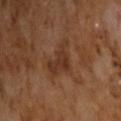workup=no biopsy performed (imaged during a skin exam) | diameter=~4 mm (longest diameter) | image-analysis metrics=a lesion area of about 7.5 mm²; a nevus-likeness score of about 0/100 and lesion-presence confidence of about 100/100 | patient=male, about 65 years old | imaging modality=total-body-photography crop, ~15 mm field of view | illumination=cross-polarized illumination.